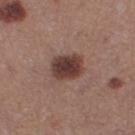The lesion was tiled from a total-body skin photograph and was not biopsied. A region of skin cropped from a whole-body photographic capture, roughly 15 mm wide. Longest diameter approximately 4 mm. The lesion is located on the right thigh. Captured under white-light illumination. The patient is a female roughly 35 years of age.